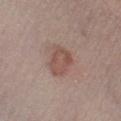Findings:
– follow-up: catalogued during a skin exam; not biopsied
– body site: the right lower leg
– image: 15 mm crop, total-body photography
– lighting: white-light illumination
– lesion diameter: ~4 mm (longest diameter)
– TBP lesion metrics: an area of roughly 9 mm² and a symmetry-axis asymmetry near 0.25; a lesion–skin lightness drop of about 8 and a normalized border contrast of about 6.5; an automated nevus-likeness rating near 55 out of 100 and a detector confidence of about 100 out of 100 that the crop contains a lesion
– subject: male, aged 53 to 57